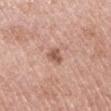Assessment: Imaged during a routine full-body skin examination; the lesion was not biopsied and no histopathology is available. Clinical summary: Approximately 2.5 mm at its widest. The lesion is on the right upper arm. A female patient, approximately 65 years of age. A 15 mm close-up tile from a total-body photography series done for melanoma screening. The tile uses white-light illumination. Automated image analysis of the tile measured a lesion area of about 3.5 mm² and an outline eccentricity of about 0.75 (0 = round, 1 = elongated). And it measured about 12 CIELAB-L* units darker than the surrounding skin.